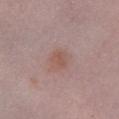Assessment:
Recorded during total-body skin imaging; not selected for excision or biopsy.